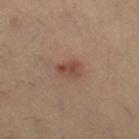Clinical impression: This lesion was catalogued during total-body skin photography and was not selected for biopsy. Image and clinical context: A 15 mm close-up extracted from a 3D total-body photography capture. The patient is a female aged 33 to 37. From the right thigh.